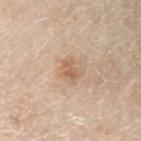Q: How was this image acquired?
A: 15 mm crop, total-body photography
Q: Where on the body is the lesion?
A: the left upper arm
Q: Patient demographics?
A: male, in their 80s
Q: What is the lesion's diameter?
A: about 2.5 mm
Q: What did automated image analysis measure?
A: an outline eccentricity of about 0.75 (0 = round, 1 = elongated) and a shape-asymmetry score of about 0.4 (0 = symmetric)